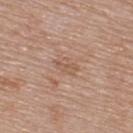| field | value |
|---|---|
| biopsy status | no biopsy performed (imaged during a skin exam) |
| subject | male, about 75 years old |
| size | about 3 mm |
| tile lighting | white-light illumination |
| anatomic site | the upper back |
| acquisition | total-body-photography crop, ~15 mm field of view |